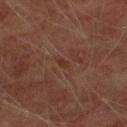Clinical impression:
No biopsy was performed on this lesion — it was imaged during a full skin examination and was not determined to be concerning.
Background:
Captured under cross-polarized illumination. A male patient approximately 75 years of age. This image is a 15 mm lesion crop taken from a total-body photograph. The lesion is located on the right lower leg.